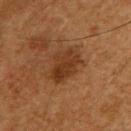biopsy_status: not biopsied; imaged during a skin examination
site: upper back
lesion_size:
  long_diameter_mm_approx: 4.0
lighting: cross-polarized
patient:
  sex: male
  age_approx: 65
image:
  source: total-body photography crop
  field_of_view_mm: 15